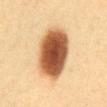follow-up — imaged on a skin check; not biopsied
location — the front of the torso
acquisition — ~15 mm tile from a whole-body skin photo
lesion size — ~7.5 mm (longest diameter)
subject — female, in their 40s
illumination — cross-polarized illumination